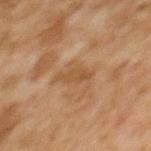Imaged during a routine full-body skin examination; the lesion was not biopsied and no histopathology is available.